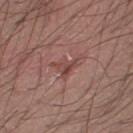The lesion was tiled from a total-body skin photograph and was not biopsied. Measured at roughly 3.5 mm in maximum diameter. The tile uses white-light illumination. A male patient aged 53–57. Automated tile analysis of the lesion measured a lesion area of about 5.5 mm² and two-axis asymmetry of about 0.55. The analysis additionally found a mean CIELAB color near L≈46 a*≈22 b*≈23 and about 7 CIELAB-L* units darker than the surrounding skin. The software also gave a border-irregularity rating of about 6.5/10, internal color variation of about 6 on a 0–10 scale, and a peripheral color-asymmetry measure near 2.5. A roughly 15 mm field-of-view crop from a total-body skin photograph.